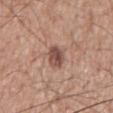<lesion>
  <image>
    <source>total-body photography crop</source>
    <field_of_view_mm>15</field_of_view_mm>
  </image>
  <site>mid back</site>
  <patient>
    <sex>male</sex>
    <age_approx>60</age_approx>
  </patient>
  <lighting>white-light</lighting>
</lesion>